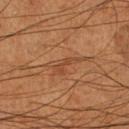Notes:
• follow-up · catalogued during a skin exam; not biopsied